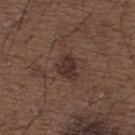This lesion was catalogued during total-body skin photography and was not selected for biopsy.
The lesion is located on the upper back.
A 15 mm close-up extracted from a 3D total-body photography capture.
The tile uses white-light illumination.
A male subject in their 50s.